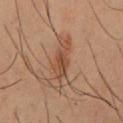Q: Was this lesion biopsied?
A: no biopsy performed (imaged during a skin exam)
Q: Who is the patient?
A: male, in their 40s
Q: What is the anatomic site?
A: the back
Q: What lighting was used for the tile?
A: cross-polarized
Q: Lesion size?
A: ~4.5 mm (longest diameter)
Q: What is the imaging modality?
A: total-body-photography crop, ~15 mm field of view